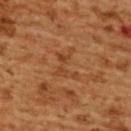Clinical impression:
The lesion was tiled from a total-body skin photograph and was not biopsied.
Clinical summary:
A close-up tile cropped from a whole-body skin photograph, about 15 mm across. From the upper back. A female patient, in their mid-50s. Imaged with cross-polarized lighting. The recorded lesion diameter is about 4 mm.